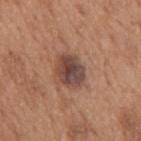* TBP lesion metrics · an average lesion color of about L≈44 a*≈20 b*≈24 (CIELAB), a lesion–skin lightness drop of about 13, and a normalized border contrast of about 10.5
* anatomic site · the upper back
* subject · male, aged 63 to 67
* acquisition · total-body-photography crop, ~15 mm field of view
* diameter · ~4 mm (longest diameter)
* tile lighting · white-light